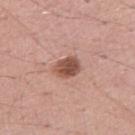Impression:
Recorded during total-body skin imaging; not selected for excision or biopsy.
Background:
A male subject, in their 50s. The lesion-visualizer software estimated a footprint of about 6 mm², an outline eccentricity of about 0.7 (0 = round, 1 = elongated), and a shape-asymmetry score of about 0.2 (0 = symmetric). And it measured a lesion color around L≈51 a*≈23 b*≈27 in CIELAB and a normalized lesion–skin contrast near 10. The software also gave a border-irregularity index near 2/10 and internal color variation of about 4 on a 0–10 scale. And it measured a lesion-detection confidence of about 100/100. Cropped from a whole-body photographic skin survey; the tile spans about 15 mm. From the arm. Approximately 3 mm at its widest. This is a white-light tile.